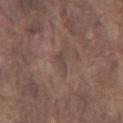Captured during whole-body skin photography for melanoma surveillance; the lesion was not biopsied. Cropped from a whole-body photographic skin survey; the tile spans about 15 mm. Located on the chest. A male subject, about 75 years old. The tile uses white-light illumination. About 2.5 mm across.